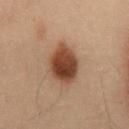workup = total-body-photography surveillance lesion; no biopsy
automated lesion analysis = a nevus-likeness score of about 100/100 and a lesion-detection confidence of about 100/100
lighting = cross-polarized illumination
subject = male, aged 53–57
diameter = ~5 mm (longest diameter)
location = the front of the torso
imaging modality = ~15 mm tile from a whole-body skin photo